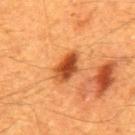workup: catalogued during a skin exam; not biopsied | subject: male, roughly 60 years of age | body site: the mid back | image: total-body-photography crop, ~15 mm field of view.